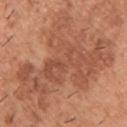workup: total-body-photography surveillance lesion; no biopsy | patient: male, aged around 40 | diameter: ~12.5 mm (longest diameter) | image: ~15 mm tile from a whole-body skin photo | tile lighting: white-light illumination | anatomic site: the right upper arm.